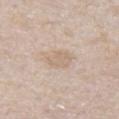Case summary:
• notes · imaged on a skin check; not biopsied
• image-analysis metrics · a mean CIELAB color near L≈66 a*≈13 b*≈28, roughly 6 lightness units darker than nearby skin, and a normalized lesion–skin contrast near 4.5; a classifier nevus-likeness of about 0/100 and a detector confidence of about 95 out of 100 that the crop contains a lesion
• acquisition · total-body-photography crop, ~15 mm field of view
• patient · male, aged around 65
• lesion size · ~2.5 mm (longest diameter)
• location · the abdomen